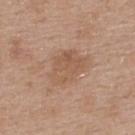Q: Was this lesion biopsied?
A: catalogued during a skin exam; not biopsied
Q: How was this image acquired?
A: 15 mm crop, total-body photography
Q: How large is the lesion?
A: ≈5 mm
Q: What did automated image analysis measure?
A: a lesion area of about 12 mm² and two-axis asymmetry of about 0.25
Q: Who is the patient?
A: female, about 40 years old
Q: Where on the body is the lesion?
A: the upper back